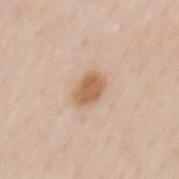No biopsy was performed on this lesion — it was imaged during a full skin examination and was not determined to be concerning. The tile uses white-light illumination. Measured at roughly 3.5 mm in maximum diameter. A female patient aged around 60. From the back. A region of skin cropped from a whole-body photographic capture, roughly 15 mm wide.